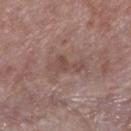Case summary:
• follow-up — catalogued during a skin exam; not biopsied
• location — the left lower leg
• acquisition — 15 mm crop, total-body photography
• automated metrics — a lesion color around L≈47 a*≈19 b*≈22 in CIELAB, roughly 8 lightness units darker than nearby skin, and a normalized border contrast of about 6; a border-irregularity index near 6/10 and a within-lesion color-variation index near 2/10; a classifier nevus-likeness of about 0/100 and a lesion-detection confidence of about 100/100
• size — about 5 mm
• subject — male, roughly 55 years of age